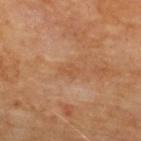Captured during whole-body skin photography for melanoma surveillance; the lesion was not biopsied. A male patient aged 68–72. Automated image analysis of the tile measured a footprint of about 3 mm², an outline eccentricity of about 0.8 (0 = round, 1 = elongated), and a symmetry-axis asymmetry near 0.3. It also reported a mean CIELAB color near L≈52 a*≈22 b*≈35. It also reported border irregularity of about 3 on a 0–10 scale, a within-lesion color-variation index near 1/10, and radial color variation of about 0.5. The tile uses cross-polarized illumination. From the upper back. The recorded lesion diameter is about 2.5 mm. Cropped from a whole-body photographic skin survey; the tile spans about 15 mm.